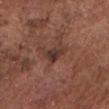Imaged during a routine full-body skin examination; the lesion was not biopsied and no histopathology is available.
This is a white-light tile.
Longest diameter approximately 2.5 mm.
Located on the head or neck.
A male patient in their mid-70s.
A 15 mm close-up tile from a total-body photography series done for melanoma screening.